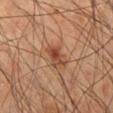Case summary:
• notes · catalogued during a skin exam; not biopsied
• automated metrics · a shape eccentricity near 0.75 and two-axis asymmetry of about 0.35; border irregularity of about 3.5 on a 0–10 scale and radial color variation of about 2
• subject · male, in their 50s
• diameter · ~3 mm (longest diameter)
• anatomic site · the right lower leg
• imaging modality · ~15 mm crop, total-body skin-cancer survey
• illumination · cross-polarized